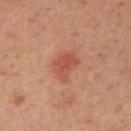Image and clinical context: On the left arm. Measured at roughly 3.5 mm in maximum diameter. A female patient, aged approximately 40. Cropped from a whole-body photographic skin survey; the tile spans about 15 mm.